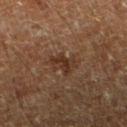- workup — catalogued during a skin exam; not biopsied